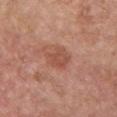The lesion was tiled from a total-body skin photograph and was not biopsied. A roughly 15 mm field-of-view crop from a total-body skin photograph. About 3.5 mm across. Imaged with white-light lighting. From the front of the torso. The patient is a female roughly 40 years of age.